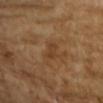This lesion was catalogued during total-body skin photography and was not selected for biopsy.
The subject is a female about 70 years old.
This image is a 15 mm lesion crop taken from a total-body photograph.
Approximately 2.5 mm at its widest.
The lesion is on the arm.
Captured under cross-polarized illumination.